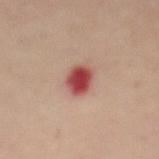notes=total-body-photography surveillance lesion; no biopsy
lesion size=about 3.5 mm
acquisition=15 mm crop, total-body photography
body site=the abdomen
illumination=cross-polarized
patient=female, in their mid-40s